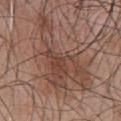<record>
  <biopsy_status>not biopsied; imaged during a skin examination</biopsy_status>
  <image>
    <source>total-body photography crop</source>
    <field_of_view_mm>15</field_of_view_mm>
  </image>
  <site>chest</site>
  <lighting>white-light</lighting>
  <automated_metrics>
    <area_mm2_approx>32.0</area_mm2_approx>
    <cielab_L>45</cielab_L>
    <cielab_a>19</cielab_a>
    <cielab_b>25</cielab_b>
    <vs_skin_contrast_norm>6.5</vs_skin_contrast_norm>
    <border_irregularity_0_10>10.0</border_irregularity_0_10>
    <color_variation_0_10>5.0</color_variation_0_10>
    <peripheral_color_asymmetry>1.5</peripheral_color_asymmetry>
  </automated_metrics>
  <patient>
    <sex>male</sex>
    <age_approx>45</age_approx>
  </patient>
  <lesion_size>
    <long_diameter_mm_approx>10.5</long_diameter_mm_approx>
  </lesion_size>
</record>